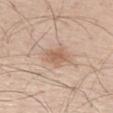This lesion was catalogued during total-body skin photography and was not selected for biopsy. Automated tile analysis of the lesion measured a mean CIELAB color near L≈61 a*≈18 b*≈31 and a lesion–skin lightness drop of about 9. The software also gave a lesion-detection confidence of about 100/100. The recorded lesion diameter is about 3.5 mm. Cropped from a whole-body photographic skin survey; the tile spans about 15 mm. From the leg. The subject is a male about 60 years old. Imaged with white-light lighting.